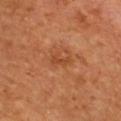Imaged during a routine full-body skin examination; the lesion was not biopsied and no histopathology is available.
Imaged with cross-polarized lighting.
The recorded lesion diameter is about 2.5 mm.
The lesion is on the chest.
A 15 mm close-up tile from a total-body photography series done for melanoma screening.
A female patient, in their mid-60s.